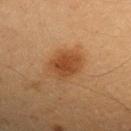Impression: Captured during whole-body skin photography for melanoma surveillance; the lesion was not biopsied. Background: The recorded lesion diameter is about 4 mm. The lesion is located on the arm. Captured under cross-polarized illumination. The lesion-visualizer software estimated a shape eccentricity near 0.6 and a shape-asymmetry score of about 0.15 (0 = symmetric). The software also gave an average lesion color of about L≈40 a*≈21 b*≈34 (CIELAB), about 8 CIELAB-L* units darker than the surrounding skin, and a normalized lesion–skin contrast near 7.5. The analysis additionally found a border-irregularity rating of about 2/10, internal color variation of about 3.5 on a 0–10 scale, and peripheral color asymmetry of about 1. And it measured a classifier nevus-likeness of about 100/100 and lesion-presence confidence of about 100/100. A 15 mm close-up extracted from a 3D total-body photography capture. The subject is a female approximately 40 years of age.